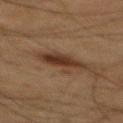No biopsy was performed on this lesion — it was imaged during a full skin examination and was not determined to be concerning. A roughly 15 mm field-of-view crop from a total-body skin photograph. About 5 mm across. This is a cross-polarized tile. The patient is a male aged around 65. An algorithmic analysis of the crop reported a shape eccentricity near 0.85. The software also gave a lesion color around L≈29 a*≈15 b*≈23 in CIELAB, roughly 9 lightness units darker than nearby skin, and a normalized border contrast of about 9. Located on the mid back.